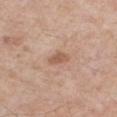follow-up: imaged on a skin check; not biopsied | tile lighting: white-light illumination | subject: male, approximately 65 years of age | location: the chest | acquisition: ~15 mm tile from a whole-body skin photo.